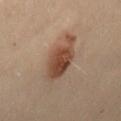workup: total-body-photography surveillance lesion; no biopsy | image: ~15 mm crop, total-body skin-cancer survey | anatomic site: the abdomen | lighting: cross-polarized | subject: female, aged around 30.